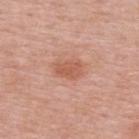Case summary:
– follow-up · imaged on a skin check; not biopsied
– body site · the upper back
– image source · total-body-photography crop, ~15 mm field of view
– lesion size · about 3 mm
– patient · female, aged 48 to 52
– lighting · white-light
– automated lesion analysis · a lesion color around L≈57 a*≈26 b*≈32 in CIELAB and a normalized border contrast of about 6.5